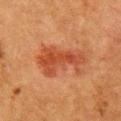biopsy_status: not biopsied; imaged during a skin examination
patient:
  sex: female
  age_approx: 50
site: front of the torso
lesion_size:
  long_diameter_mm_approx: 6.0
lighting: cross-polarized
image:
  source: total-body photography crop
  field_of_view_mm: 15